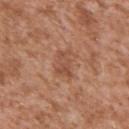The lesion was tiled from a total-body skin photograph and was not biopsied. A 15 mm close-up tile from a total-body photography series done for melanoma screening. An algorithmic analysis of the crop reported an automated nevus-likeness rating near 0 out of 100 and lesion-presence confidence of about 100/100. Measured at roughly 3.5 mm in maximum diameter. The lesion is on the left upper arm. A male subject aged 43–47. Imaged with white-light lighting.